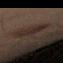Findings:
• notes: imaged on a skin check; not biopsied
• size: about 3.5 mm
• acquisition: total-body-photography crop, ~15 mm field of view
• lighting: cross-polarized illumination
• subject: male, roughly 45 years of age
• body site: the leg